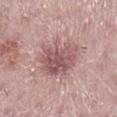biopsy status = no biopsy performed (imaged during a skin exam).